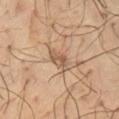Clinical impression: Captured during whole-body skin photography for melanoma surveillance; the lesion was not biopsied. Acquisition and patient details: Measured at roughly 3 mm in maximum diameter. Located on the abdomen. A region of skin cropped from a whole-body photographic capture, roughly 15 mm wide. A female patient, aged 48 to 52.